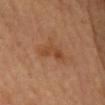Q: Patient demographics?
A: female, roughly 60 years of age
Q: What is the imaging modality?
A: 15 mm crop, total-body photography
Q: What is the anatomic site?
A: the mid back